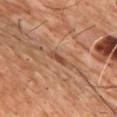{"biopsy_status": "not biopsied; imaged during a skin examination", "patient": {"sex": "male", "age_approx": 65}, "automated_metrics": {"area_mm2_approx": 2.5, "eccentricity": 0.95, "shape_asymmetry": 0.25, "border_irregularity_0_10": 2.5, "peripheral_color_asymmetry": 0.0}, "lesion_size": {"long_diameter_mm_approx": 3.0}, "image": {"source": "total-body photography crop", "field_of_view_mm": 15}, "lighting": "cross-polarized", "site": "chest"}